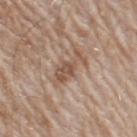{
  "biopsy_status": "not biopsied; imaged during a skin examination",
  "lesion_size": {
    "long_diameter_mm_approx": 4.5
  },
  "patient": {
    "sex": "male",
    "age_approx": 60
  },
  "site": "left upper arm",
  "image": {
    "source": "total-body photography crop",
    "field_of_view_mm": 15
  },
  "automated_metrics": {
    "border_irregularity_0_10": 7.0,
    "color_variation_0_10": 2.5,
    "peripheral_color_asymmetry": 1.0
  }
}